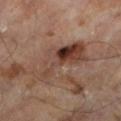Imaged during a routine full-body skin examination; the lesion was not biopsied and no histopathology is available. Automated image analysis of the tile measured a lesion color around L≈40 a*≈18 b*≈25 in CIELAB and a lesion–skin lightness drop of about 10. The software also gave a nevus-likeness score of about 0/100 and a detector confidence of about 100 out of 100 that the crop contains a lesion. A 15 mm crop from a total-body photograph taken for skin-cancer surveillance. Measured at roughly 7.5 mm in maximum diameter. Captured under cross-polarized illumination. The lesion is on the left lower leg. A male patient aged around 65.